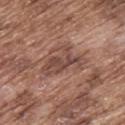Impression:
This lesion was catalogued during total-body skin photography and was not selected for biopsy.
Acquisition and patient details:
A 15 mm close-up extracted from a 3D total-body photography capture. A male subject, aged 73–77. This is a white-light tile. The lesion is on the upper back.